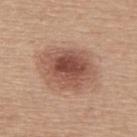biopsy status: total-body-photography surveillance lesion; no biopsy
image source: 15 mm crop, total-body photography
anatomic site: the upper back
lesion diameter: about 6 mm
subject: female, aged 63 to 67